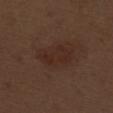patient:
  sex: male
  age_approx: 70
site: right thigh
image:
  source: total-body photography crop
  field_of_view_mm: 15
lesion_size:
  long_diameter_mm_approx: 4.5
lighting: white-light